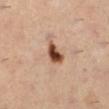The lesion is located on the left lower leg. Cropped from a total-body skin-imaging series; the visible field is about 15 mm. Approximately 3 mm at its widest. The subject is a female aged 33–37. An algorithmic analysis of the crop reported a footprint of about 5 mm² and an outline eccentricity of about 0.7 (0 = round, 1 = elongated). And it measured a mean CIELAB color near L≈45 a*≈22 b*≈31, a lesion–skin lightness drop of about 19, and a lesion-to-skin contrast of about 13.5 (normalized; higher = more distinct). And it measured border irregularity of about 2.5 on a 0–10 scale. The analysis additionally found a classifier nevus-likeness of about 100/100 and a detector confidence of about 100 out of 100 that the crop contains a lesion. Imaged with cross-polarized lighting. Histopathological examination showed a benign skin lesion: junctional melanocytic nevus.